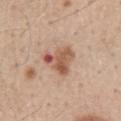Background: About 4 mm across. Cropped from a total-body skin-imaging series; the visible field is about 15 mm. A male patient approximately 60 years of age. Located on the lower back. The tile uses white-light illumination.